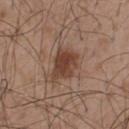{
  "biopsy_status": "not biopsied; imaged during a skin examination",
  "image": {
    "source": "total-body photography crop",
    "field_of_view_mm": 15
  },
  "patient": {
    "sex": "male",
    "age_approx": 50
  },
  "lesion_size": {
    "long_diameter_mm_approx": 4.0
  },
  "lighting": "white-light",
  "site": "upper back"
}